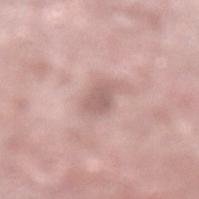workup = catalogued during a skin exam; not biopsied | automated lesion analysis = an average lesion color of about L≈59 a*≈19 b*≈22 (CIELAB), roughly 10 lightness units darker than nearby skin, and a normalized lesion–skin contrast near 6.5; an automated nevus-likeness rating near 0 out of 100 and a lesion-detection confidence of about 100/100 | lighting = white-light | location = the left lower leg | acquisition = total-body-photography crop, ~15 mm field of view | patient = male, roughly 75 years of age.